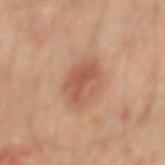Image and clinical context:
The patient is a male aged 63–67. A 15 mm close-up tile from a total-body photography series done for melanoma screening. About 4 mm across. The lesion is on the mid back.